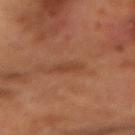Q: Is there a histopathology result?
A: imaged on a skin check; not biopsied
Q: What are the patient's age and sex?
A: male, approximately 65 years of age
Q: What is the lesion's diameter?
A: about 2.5 mm
Q: What lighting was used for the tile?
A: cross-polarized illumination
Q: What kind of image is this?
A: ~15 mm tile from a whole-body skin photo
Q: What did automated image analysis measure?
A: peripheral color asymmetry of about 0; a classifier nevus-likeness of about 0/100 and lesion-presence confidence of about 100/100